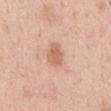Captured during whole-body skin photography for melanoma surveillance; the lesion was not biopsied.
A female subject roughly 50 years of age.
On the mid back.
Cropped from a whole-body photographic skin survey; the tile spans about 15 mm.